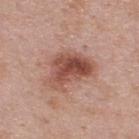biopsy_status: not biopsied; imaged during a skin examination
patient:
  sex: male
  age_approx: 45
lesion_size:
  long_diameter_mm_approx: 6.0
image:
  source: total-body photography crop
  field_of_view_mm: 15
lighting: white-light
site: back
automated_metrics:
  area_mm2_approx: 16.0
  eccentricity: 0.75
  shape_asymmetry: 0.45